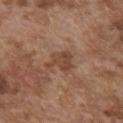Imaged during a routine full-body skin examination; the lesion was not biopsied and no histopathology is available.
Captured under white-light illumination.
The subject is a male aged 73–77.
The lesion is on the front of the torso.
A 15 mm close-up tile from a total-body photography series done for melanoma screening.